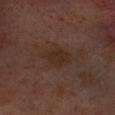Q: Was a biopsy performed?
A: total-body-photography surveillance lesion; no biopsy
Q: Patient demographics?
A: female, aged approximately 55
Q: What is the imaging modality?
A: 15 mm crop, total-body photography
Q: Lesion location?
A: the head or neck
Q: How large is the lesion?
A: about 2.5 mm
Q: Illumination type?
A: cross-polarized illumination
Q: What did automated image analysis measure?
A: a lesion area of about 5.5 mm² and a symmetry-axis asymmetry near 0.15; a nevus-likeness score of about 0/100